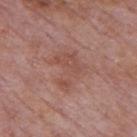Assessment: The lesion was tiled from a total-body skin photograph and was not biopsied. Clinical summary: Imaged with white-light lighting. A male patient, about 75 years old. Located on the mid back. A close-up tile cropped from a whole-body skin photograph, about 15 mm across. Measured at roughly 4.5 mm in maximum diameter.